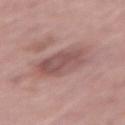The lesion was tiled from a total-body skin photograph and was not biopsied. Automated tile analysis of the lesion measured a lesion area of about 17 mm² and two-axis asymmetry of about 0.15. The analysis additionally found a lesion–skin lightness drop of about 9. The software also gave a border-irregularity index near 1.5/10, internal color variation of about 4 on a 0–10 scale, and a peripheral color-asymmetry measure near 1.5. It also reported a nevus-likeness score of about 0/100 and a lesion-detection confidence of about 100/100. A male patient, aged 68 to 72. Approximately 5.5 mm at its widest. Imaged with white-light lighting. The lesion is on the leg. A close-up tile cropped from a whole-body skin photograph, about 15 mm across.